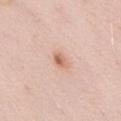biopsy status: total-body-photography surveillance lesion; no biopsy | image: total-body-photography crop, ~15 mm field of view | tile lighting: white-light illumination | subject: male, aged approximately 55 | anatomic site: the chest.